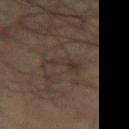Captured during whole-body skin photography for melanoma surveillance; the lesion was not biopsied. Measured at roughly 3.5 mm in maximum diameter. The lesion is located on the right thigh. This is a cross-polarized tile. A 15 mm close-up extracted from a 3D total-body photography capture. The total-body-photography lesion software estimated an area of roughly 5 mm² and two-axis asymmetry of about 0.65. The software also gave a mean CIELAB color near L≈25 a*≈8 b*≈16, roughly 5 lightness units darker than nearby skin, and a lesion-to-skin contrast of about 5.5 (normalized; higher = more distinct). And it measured internal color variation of about 1.5 on a 0–10 scale and a peripheral color-asymmetry measure near 0.5. A male patient aged approximately 70.